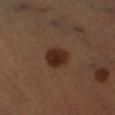Clinical impression: The lesion was tiled from a total-body skin photograph and was not biopsied. Background: Located on the left lower leg. Approximately 3 mm at its widest. A close-up tile cropped from a whole-body skin photograph, about 15 mm across. The tile uses cross-polarized illumination. Automated image analysis of the tile measured an area of roughly 7 mm², a shape eccentricity near 0.55, and a shape-asymmetry score of about 0.15 (0 = symmetric). It also reported an average lesion color of about L≈27 a*≈18 b*≈26 (CIELAB), a lesion–skin lightness drop of about 10, and a normalized border contrast of about 10.5. The analysis additionally found radial color variation of about 1. The analysis additionally found a classifier nevus-likeness of about 100/100. The patient is a male in their 50s.